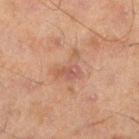Assessment:
No biopsy was performed on this lesion — it was imaged during a full skin examination and was not determined to be concerning.
Background:
A male subject, aged approximately 45. A 15 mm close-up extracted from a 3D total-body photography capture. Measured at roughly 4 mm in maximum diameter. From the left lower leg. Imaged with cross-polarized lighting.